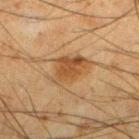notes — catalogued during a skin exam; not biopsied
tile lighting — cross-polarized illumination
image source — ~15 mm crop, total-body skin-cancer survey
size — about 5 mm
image-analysis metrics — a mean CIELAB color near L≈39 a*≈16 b*≈32, a lesion–skin lightness drop of about 8, and a normalized lesion–skin contrast near 7.5; a border-irregularity rating of about 3.5/10 and a peripheral color-asymmetry measure near 1
subject — male, about 35 years old
site — the left lower leg